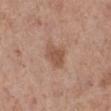No biopsy was performed on this lesion — it was imaged during a full skin examination and was not determined to be concerning.
A female subject, aged approximately 40.
On the right lower leg.
A 15 mm close-up tile from a total-body photography series done for melanoma screening.
The total-body-photography lesion software estimated a footprint of about 6.5 mm² and a shape-asymmetry score of about 0.3 (0 = symmetric). And it measured an average lesion color of about L≈53 a*≈21 b*≈29 (CIELAB) and roughly 9 lightness units darker than nearby skin. It also reported an automated nevus-likeness rating near 35 out of 100.
This is a white-light tile.